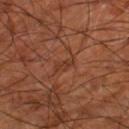Imaged during a routine full-body skin examination; the lesion was not biopsied and no histopathology is available. On the leg. This image is a 15 mm lesion crop taken from a total-body photograph. The subject is about 65 years old.